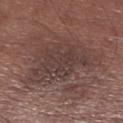Assessment: Recorded during total-body skin imaging; not selected for excision or biopsy. Image and clinical context: This is a white-light tile. Automated tile analysis of the lesion measured a classifier nevus-likeness of about 0/100 and a lesion-detection confidence of about 85/100. A lesion tile, about 15 mm wide, cut from a 3D total-body photograph. The subject is a male roughly 55 years of age. The lesion's longest dimension is about 9 mm. Located on the leg.